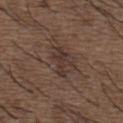Case summary:
* biopsy status — imaged on a skin check; not biopsied
* subject — male, roughly 50 years of age
* lighting — white-light illumination
* image source — 15 mm crop, total-body photography
* site — the back